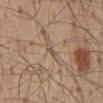Imaged during a routine full-body skin examination; the lesion was not biopsied and no histopathology is available. The lesion-visualizer software estimated a footprint of about 1 mm², an eccentricity of roughly 0.75, and two-axis asymmetry of about 0.35. It also reported a border-irregularity rating of about 2.5/10, a color-variation rating of about 0/10, and a peripheral color-asymmetry measure near 0. It also reported a nevus-likeness score of about 0/100 and a lesion-detection confidence of about 0/100. A male patient, in their mid-50s. The lesion is located on the back. Longest diameter approximately 1 mm. A 15 mm close-up tile from a total-body photography series done for melanoma screening. This is a white-light tile.